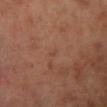Q: Is there a histopathology result?
A: total-body-photography surveillance lesion; no biopsy
Q: What kind of image is this?
A: ~15 mm crop, total-body skin-cancer survey
Q: What lighting was used for the tile?
A: cross-polarized illumination
Q: What are the patient's age and sex?
A: male, about 70 years old
Q: Where on the body is the lesion?
A: the left lower leg
Q: Lesion size?
A: ≈1 mm